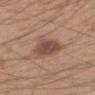Notes:
* follow-up — no biopsy performed (imaged during a skin exam)
* illumination — white-light
* subject — male, aged around 20
* anatomic site — the left forearm
* image source — 15 mm crop, total-body photography
* automated metrics — an area of roughly 11 mm², an eccentricity of roughly 0.2, and two-axis asymmetry of about 0.25; a classifier nevus-likeness of about 50/100 and a detector confidence of about 100 out of 100 that the crop contains a lesion